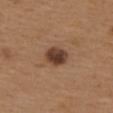Part of a total-body skin-imaging series; this lesion was reviewed on a skin check and was not flagged for biopsy. The lesion is located on the back. A female subject aged approximately 40. An algorithmic analysis of the crop reported a lesion color around L≈40 a*≈19 b*≈27 in CIELAB, a lesion–skin lightness drop of about 14, and a lesion-to-skin contrast of about 11 (normalized; higher = more distinct). It also reported a color-variation rating of about 5/10 and radial color variation of about 1.5. And it measured a classifier nevus-likeness of about 80/100 and a lesion-detection confidence of about 100/100. Longest diameter approximately 3.5 mm. Captured under white-light illumination. This image is a 15 mm lesion crop taken from a total-body photograph.